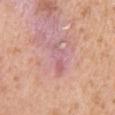{
  "biopsy_status": "not biopsied; imaged during a skin examination",
  "lighting": "white-light",
  "lesion_size": {
    "long_diameter_mm_approx": 4.5
  },
  "site": "right upper arm",
  "automated_metrics": {
    "border_irregularity_0_10": 6.0,
    "color_variation_0_10": 4.5
  },
  "image": {
    "source": "total-body photography crop",
    "field_of_view_mm": 15
  },
  "patient": {
    "sex": "female",
    "age_approx": 40
  }
}